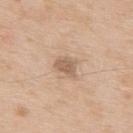Context:
The lesion's longest dimension is about 2.5 mm. This image is a 15 mm lesion crop taken from a total-body photograph. The lesion is located on the upper back. A male patient, in their mid- to late 60s.